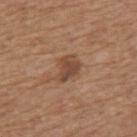No biopsy was performed on this lesion — it was imaged during a full skin examination and was not determined to be concerning. A region of skin cropped from a whole-body photographic capture, roughly 15 mm wide. This is a white-light tile. The subject is a male aged around 65. From the mid back. Approximately 3.5 mm at its widest.